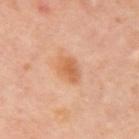No biopsy was performed on this lesion — it was imaged during a full skin examination and was not determined to be concerning.